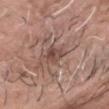The lesion was photographed on a routine skin check and not biopsied; there is no pathology result. This is a white-light tile. Measured at roughly 3 mm in maximum diameter. A 15 mm close-up extracted from a 3D total-body photography capture. A male patient approximately 70 years of age. The lesion is on the head or neck.